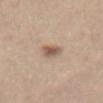{"biopsy_status": "not biopsied; imaged during a skin examination", "lighting": "white-light", "image": {"source": "total-body photography crop", "field_of_view_mm": 15}, "patient": {"sex": "female", "age_approx": 45}, "lesion_size": {"long_diameter_mm_approx": 3.0}, "site": "front of the torso"}